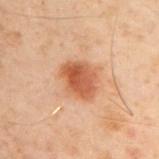| key | value |
|---|---|
| notes | imaged on a skin check; not biopsied |
| lighting | cross-polarized |
| diameter | ~4.5 mm (longest diameter) |
| body site | the upper back |
| acquisition | ~15 mm crop, total-body skin-cancer survey |
| patient | male, aged approximately 50 |
| automated metrics | an area of roughly 11 mm² and an eccentricity of roughly 0.65; internal color variation of about 5 on a 0–10 scale and a peripheral color-asymmetry measure near 1.5 |